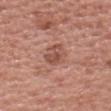Case summary:
* biopsy status · no biopsy performed (imaged during a skin exam)
* lesion diameter · about 3.5 mm
* patient · male, aged 68 to 72
* automated metrics · a shape-asymmetry score of about 0.3 (0 = symmetric); an average lesion color of about L≈51 a*≈24 b*≈28 (CIELAB), a lesion–skin lightness drop of about 9, and a lesion-to-skin contrast of about 6.5 (normalized; higher = more distinct); a border-irregularity rating of about 3.5/10, a color-variation rating of about 3.5/10, and a peripheral color-asymmetry measure near 1
* lighting · white-light illumination
* anatomic site · the head or neck
* imaging modality · ~15 mm tile from a whole-body skin photo